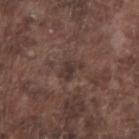biopsy status: catalogued during a skin exam; not biopsied | lighting: white-light illumination | body site: the arm | image-analysis metrics: a footprint of about 3.5 mm², a shape eccentricity near 0.6, and two-axis asymmetry of about 0.45; a lesion color around L≈33 a*≈15 b*≈18 in CIELAB, about 7 CIELAB-L* units darker than the surrounding skin, and a normalized border contrast of about 7.5; a within-lesion color-variation index near 2/10 and peripheral color asymmetry of about 0.5 | size: ~2.5 mm (longest diameter) | patient: male, approximately 75 years of age | image source: total-body-photography crop, ~15 mm field of view.